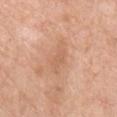No biopsy was performed on this lesion — it was imaged during a full skin examination and was not determined to be concerning. A male patient, aged 58–62. Imaged with white-light lighting. A lesion tile, about 15 mm wide, cut from a 3D total-body photograph. Measured at roughly 4.5 mm in maximum diameter. Located on the left upper arm.